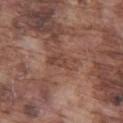notes=imaged on a skin check; not biopsied | patient=male, approximately 75 years of age | image=~15 mm crop, total-body skin-cancer survey | automated metrics=a shape eccentricity near 0.9; a nevus-likeness score of about 0/100 and lesion-presence confidence of about 90/100 | location=the left thigh | illumination=white-light.